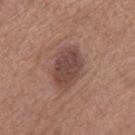Impression: The lesion was tiled from a total-body skin photograph and was not biopsied. Background: A female subject approximately 65 years of age. From the leg. A region of skin cropped from a whole-body photographic capture, roughly 15 mm wide. This is a white-light tile.